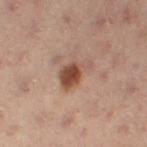| key | value |
|---|---|
| biopsy status | total-body-photography surveillance lesion; no biopsy |
| image | 15 mm crop, total-body photography |
| site | the left leg |
| subject | female, in their mid-50s |